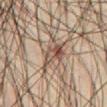Q: How was this image acquired?
A: total-body-photography crop, ~15 mm field of view
Q: Illumination type?
A: cross-polarized illumination
Q: What is the anatomic site?
A: the abdomen
Q: What did automated image analysis measure?
A: a lesion–skin lightness drop of about 10 and a normalized lesion–skin contrast near 8; a lesion-detection confidence of about 85/100
Q: Patient demographics?
A: male, approximately 60 years of age